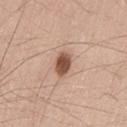Imaged during a routine full-body skin examination; the lesion was not biopsied and no histopathology is available. The total-body-photography lesion software estimated about 17 CIELAB-L* units darker than the surrounding skin and a normalized lesion–skin contrast near 11. Located on the leg. This is a white-light tile. Approximately 3 mm at its widest. A male patient aged approximately 45. Cropped from a whole-body photographic skin survey; the tile spans about 15 mm.